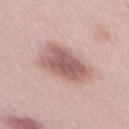Part of a total-body skin-imaging series; this lesion was reviewed on a skin check and was not flagged for biopsy. The total-body-photography lesion software estimated a footprint of about 19 mm², an eccentricity of roughly 0.8, and a shape-asymmetry score of about 0.2 (0 = symmetric). The recorded lesion diameter is about 6 mm. Captured under white-light illumination. Cropped from a total-body skin-imaging series; the visible field is about 15 mm. A male patient aged 28 to 32.